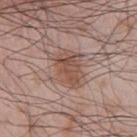Context:
Located on the front of the torso. A male subject, approximately 65 years of age. The total-body-photography lesion software estimated an area of roughly 13 mm², an outline eccentricity of about 0.8 (0 = round, 1 = elongated), and a symmetry-axis asymmetry near 0.3. This image is a 15 mm lesion crop taken from a total-body photograph. Captured under white-light illumination. Approximately 5 mm at its widest.